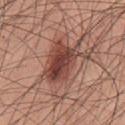Clinical impression:
The lesion was tiled from a total-body skin photograph and was not biopsied.
Clinical summary:
On the front of the torso. About 6 mm across. This is a white-light tile. A male patient approximately 60 years of age. A close-up tile cropped from a whole-body skin photograph, about 15 mm across.